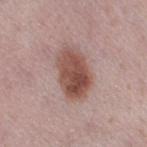biopsy status: no biopsy performed (imaged during a skin exam); image-analysis metrics: a border-irregularity index near 2/10, a within-lesion color-variation index near 6/10, and radial color variation of about 2.5; illumination: white-light illumination; subject: female, in their 40s; anatomic site: the right thigh; size: ~6 mm (longest diameter); image: ~15 mm crop, total-body skin-cancer survey.